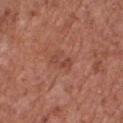biopsy status = imaged on a skin check; not biopsied | illumination = white-light illumination | acquisition = ~15 mm tile from a whole-body skin photo | subject = male, about 75 years old | site = the chest | size = ≈3 mm.